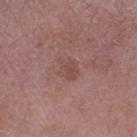Impression: This lesion was catalogued during total-body skin photography and was not selected for biopsy. Background: The tile uses white-light illumination. A female subject, in their 70s. Located on the left thigh. A lesion tile, about 15 mm wide, cut from a 3D total-body photograph.